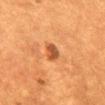biopsy status — catalogued during a skin exam; not biopsied | tile lighting — cross-polarized illumination | site — the mid back | diameter — ≈2.5 mm | image — ~15 mm tile from a whole-body skin photo | subject — female, aged 53 to 57.